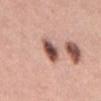Assessment: Recorded during total-body skin imaging; not selected for excision or biopsy. Acquisition and patient details: Measured at roughly 3.5 mm in maximum diameter. From the abdomen. A 15 mm close-up extracted from a 3D total-body photography capture. Imaged with white-light lighting. The patient is a male about 40 years old. The lesion-visualizer software estimated a lesion area of about 6 mm², an eccentricity of roughly 0.8, and two-axis asymmetry of about 0.2. It also reported a mean CIELAB color near L≈52 a*≈22 b*≈24, about 19 CIELAB-L* units darker than the surrounding skin, and a normalized lesion–skin contrast near 12. It also reported a classifier nevus-likeness of about 90/100 and lesion-presence confidence of about 100/100.